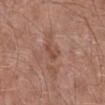follow-up = total-body-photography surveillance lesion; no biopsy
TBP lesion metrics = a lesion color around L≈48 a*≈22 b*≈27 in CIELAB, about 8 CIELAB-L* units darker than the surrounding skin, and a normalized lesion–skin contrast near 6; border irregularity of about 4.5 on a 0–10 scale, internal color variation of about 1 on a 0–10 scale, and peripheral color asymmetry of about 0.5; a classifier nevus-likeness of about 0/100 and a detector confidence of about 100 out of 100 that the crop contains a lesion
patient = male, about 55 years old
imaging modality = ~15 mm crop, total-body skin-cancer survey
location = the right lower leg
size = about 2.5 mm
tile lighting = white-light illumination